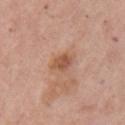Findings:
• workup — catalogued during a skin exam; not biopsied
• lighting — white-light
• subject — female, aged 63 to 67
• lesion diameter — ~2.5 mm (longest diameter)
• location — the left upper arm
• imaging modality — 15 mm crop, total-body photography